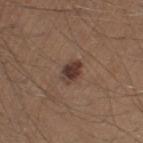The lesion was tiled from a total-body skin photograph and was not biopsied. On the left thigh. A male subject aged around 30. The total-body-photography lesion software estimated an area of roughly 4.5 mm², an eccentricity of roughly 0.7, and two-axis asymmetry of about 0.15. The software also gave a lesion color around L≈36 a*≈17 b*≈23 in CIELAB, about 12 CIELAB-L* units darker than the surrounding skin, and a normalized border contrast of about 10.5. The analysis additionally found a nevus-likeness score of about 80/100 and a lesion-detection confidence of about 100/100. Cropped from a whole-body photographic skin survey; the tile spans about 15 mm. Imaged with white-light lighting.